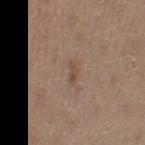From the left thigh. The recorded lesion diameter is about 2.5 mm. A 15 mm crop from a total-body photograph taken for skin-cancer surveillance. The subject is a female aged 38 to 42.